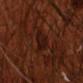Q: Is there a histopathology result?
A: total-body-photography surveillance lesion; no biopsy
Q: What is the anatomic site?
A: the right upper arm
Q: Automated lesion metrics?
A: border irregularity of about 3 on a 0–10 scale, internal color variation of about 2 on a 0–10 scale, and radial color variation of about 0.5
Q: What is the lesion's diameter?
A: about 3 mm
Q: What lighting was used for the tile?
A: cross-polarized
Q: Who is the patient?
A: male, aged approximately 70
Q: How was this image acquired?
A: total-body-photography crop, ~15 mm field of view